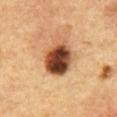biopsy status = imaged on a skin check; not biopsied | image = ~15 mm crop, total-body skin-cancer survey | size = ~5 mm (longest diameter) | illumination = cross-polarized | body site = the chest | subject = male, in their mid-60s.